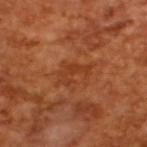| feature | finding |
|---|---|
| follow-up | imaged on a skin check; not biopsied |
| image | total-body-photography crop, ~15 mm field of view |
| subject | male, aged 63 to 67 |
| automated metrics | a lesion area of about 7.5 mm², an outline eccentricity of about 0.8 (0 = round, 1 = elongated), and a shape-asymmetry score of about 0.6 (0 = symmetric); a classifier nevus-likeness of about 0/100 |
| lesion size | about 4 mm |
| lighting | cross-polarized illumination |